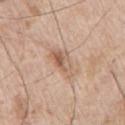{"biopsy_status": "not biopsied; imaged during a skin examination", "automated_metrics": {"area_mm2_approx": 8.0, "eccentricity": 0.95, "shape_asymmetry": 0.3, "nevus_likeness_0_100": 40, "lesion_detection_confidence_0_100": 100}, "image": {"source": "total-body photography crop", "field_of_view_mm": 15}, "site": "right upper arm", "lighting": "white-light", "patient": {"sex": "male", "age_approx": 65}}